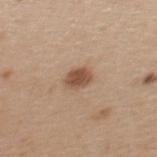The lesion was photographed on a routine skin check and not biopsied; there is no pathology result.
A close-up tile cropped from a whole-body skin photograph, about 15 mm across.
The lesion's longest dimension is about 3 mm.
The total-body-photography lesion software estimated a border-irregularity rating of about 1.5/10 and radial color variation of about 1. The software also gave a classifier nevus-likeness of about 95/100 and a lesion-detection confidence of about 100/100.
Imaged with white-light lighting.
The patient is a female aged around 45.
On the back.